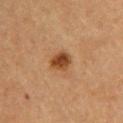The lesion was photographed on a routine skin check and not biopsied; there is no pathology result. The lesion is on the upper back. This is a cross-polarized tile. A close-up tile cropped from a whole-body skin photograph, about 15 mm across. A female subject, aged approximately 60.